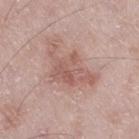Recorded during total-body skin imaging; not selected for excision or biopsy.
The tile uses white-light illumination.
An algorithmic analysis of the crop reported an area of roughly 16 mm² and a symmetry-axis asymmetry near 0.5. And it measured a border-irregularity index near 6.5/10, a within-lesion color-variation index near 4/10, and peripheral color asymmetry of about 1.
Longest diameter approximately 7 mm.
Located on the right thigh.
A roughly 15 mm field-of-view crop from a total-body skin photograph.
A male patient in their mid-60s.